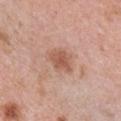Assessment:
Part of a total-body skin-imaging series; this lesion was reviewed on a skin check and was not flagged for biopsy.
Clinical summary:
This image is a 15 mm lesion crop taken from a total-body photograph. Longest diameter approximately 3.5 mm. The tile uses white-light illumination. A female subject, in their 40s. Automated image analysis of the tile measured an area of roughly 6 mm², an eccentricity of roughly 0.65, and two-axis asymmetry of about 0.25. The analysis additionally found a mean CIELAB color near L≈56 a*≈23 b*≈30, roughly 10 lightness units darker than nearby skin, and a normalized lesion–skin contrast near 7. It also reported a border-irregularity rating of about 2.5/10, internal color variation of about 2 on a 0–10 scale, and radial color variation of about 0.5. It also reported a classifier nevus-likeness of about 25/100 and a detector confidence of about 100 out of 100 that the crop contains a lesion. From the chest.